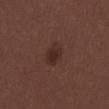{
  "patient": {
    "sex": "male",
    "age_approx": 30
  },
  "image": {
    "source": "total-body photography crop",
    "field_of_view_mm": 15
  },
  "site": "lower back"
}